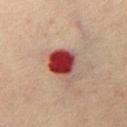Part of a total-body skin-imaging series; this lesion was reviewed on a skin check and was not flagged for biopsy. An algorithmic analysis of the crop reported an outline eccentricity of about 0.5 (0 = round, 1 = elongated) and a symmetry-axis asymmetry near 0.25. The analysis additionally found an average lesion color of about L≈35 a*≈28 b*≈23 (CIELAB), a lesion–skin lightness drop of about 17, and a normalized border contrast of about 14. The software also gave border irregularity of about 2.5 on a 0–10 scale, internal color variation of about 8.5 on a 0–10 scale, and peripheral color asymmetry of about 2.5. And it measured a classifier nevus-likeness of about 0/100 and a lesion-detection confidence of about 100/100. The subject is a male aged 68–72. Located on the mid back. A 15 mm close-up extracted from a 3D total-body photography capture. The recorded lesion diameter is about 4 mm. This is a cross-polarized tile.